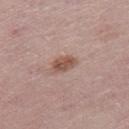biopsy status — catalogued during a skin exam; not biopsied
subject — female, in their 50s
site — the leg
lesion diameter — ≈3 mm
imaging modality — 15 mm crop, total-body photography